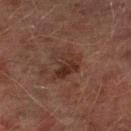Assessment: The lesion was photographed on a routine skin check and not biopsied; there is no pathology result. Clinical summary: Longest diameter approximately 3.5 mm. From the left lower leg. Captured under cross-polarized illumination. Cropped from a whole-body photographic skin survey; the tile spans about 15 mm. A male subject about 75 years old. An algorithmic analysis of the crop reported a lesion area of about 9 mm² and a shape eccentricity near 0.6. The analysis additionally found a border-irregularity index near 5/10, internal color variation of about 5.5 on a 0–10 scale, and peripheral color asymmetry of about 2.